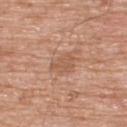{
  "biopsy_status": "not biopsied; imaged during a skin examination",
  "image": {
    "source": "total-body photography crop",
    "field_of_view_mm": 15
  },
  "site": "upper back",
  "lesion_size": {
    "long_diameter_mm_approx": 2.5
  },
  "patient": {
    "sex": "male",
    "age_approx": 60
  },
  "lighting": "white-light"
}